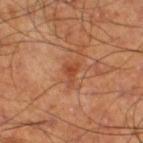Imaged during a routine full-body skin examination; the lesion was not biopsied and no histopathology is available. The patient is a male about 60 years old. The lesion is located on the leg. This image is a 15 mm lesion crop taken from a total-body photograph.